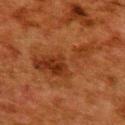Part of a total-body skin-imaging series; this lesion was reviewed on a skin check and was not flagged for biopsy.